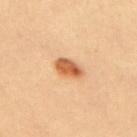Clinical impression: No biopsy was performed on this lesion — it was imaged during a full skin examination and was not determined to be concerning. Clinical summary: The lesion is on the upper back. A female patient roughly 35 years of age. Approximately 3.5 mm at its widest. The tile uses cross-polarized illumination. A 15 mm close-up extracted from a 3D total-body photography capture.